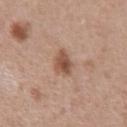tile lighting: white-light illumination; size: ~3 mm (longest diameter); subject: male, aged approximately 55; anatomic site: the chest; acquisition: total-body-photography crop, ~15 mm field of view.